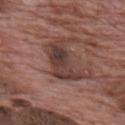Assessment:
No biopsy was performed on this lesion — it was imaged during a full skin examination and was not determined to be concerning.
Image and clinical context:
A 15 mm close-up tile from a total-body photography series done for melanoma screening. A male patient, aged approximately 70. This is a white-light tile. The lesion is on the upper back.